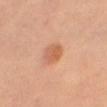Part of a total-body skin-imaging series; this lesion was reviewed on a skin check and was not flagged for biopsy.
On the right thigh.
Imaged with cross-polarized lighting.
The recorded lesion diameter is about 3 mm.
A 15 mm crop from a total-body photograph taken for skin-cancer surveillance.
A female patient, aged 28 to 32.